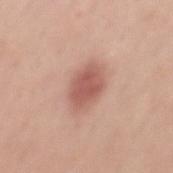Clinical impression: Captured during whole-body skin photography for melanoma surveillance; the lesion was not biopsied. Clinical summary: A close-up tile cropped from a whole-body skin photograph, about 15 mm across. Captured under white-light illumination. On the abdomen. The total-body-photography lesion software estimated a border-irregularity index near 2/10, a within-lesion color-variation index near 2.5/10, and radial color variation of about 0.5. Approximately 5 mm at its widest. The patient is a female roughly 35 years of age.